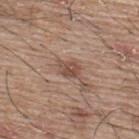workup: no biopsy performed (imaged during a skin exam) | location: the upper back | acquisition: ~15 mm crop, total-body skin-cancer survey | subject: male, aged approximately 65 | lighting: white-light illumination | automated lesion analysis: border irregularity of about 3.5 on a 0–10 scale, internal color variation of about 3 on a 0–10 scale, and radial color variation of about 1; a detector confidence of about 100 out of 100 that the crop contains a lesion | size: about 3 mm.